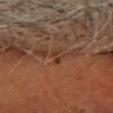No biopsy was performed on this lesion — it was imaged during a full skin examination and was not determined to be concerning. Cropped from a whole-body photographic skin survey; the tile spans about 15 mm. On the head or neck. The tile uses cross-polarized illumination. A female subject aged 78–82. The recorded lesion diameter is about 3 mm.